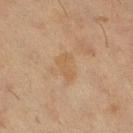Part of a total-body skin-imaging series; this lesion was reviewed on a skin check and was not flagged for biopsy. A female subject aged 53 to 57. Located on the right lower leg. This image is a 15 mm lesion crop taken from a total-body photograph. The recorded lesion diameter is about 3 mm. The lesion-visualizer software estimated a lesion area of about 4.5 mm², an eccentricity of roughly 0.85, and a symmetry-axis asymmetry near 0.45. The software also gave a lesion color around L≈50 a*≈15 b*≈32 in CIELAB, a lesion–skin lightness drop of about 5, and a normalized lesion–skin contrast near 5.